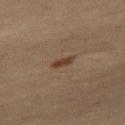• notes — catalogued during a skin exam; not biopsied